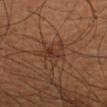Notes:
– follow-up: total-body-photography surveillance lesion; no biopsy
– lesion diameter: about 4 mm
– image: ~15 mm tile from a whole-body skin photo
– tile lighting: cross-polarized illumination
– TBP lesion metrics: an area of roughly 6.5 mm², an outline eccentricity of about 0.75 (0 = round, 1 = elongated), and a symmetry-axis asymmetry near 0.4; a classifier nevus-likeness of about 0/100 and a detector confidence of about 100 out of 100 that the crop contains a lesion
– subject: male, aged 53 to 57
– anatomic site: the arm